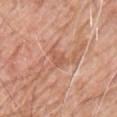Assessment: No biopsy was performed on this lesion — it was imaged during a full skin examination and was not determined to be concerning. Background: An algorithmic analysis of the crop reported an area of roughly 4.5 mm², an eccentricity of roughly 0.75, and a symmetry-axis asymmetry near 0.45. The software also gave border irregularity of about 4.5 on a 0–10 scale, internal color variation of about 2 on a 0–10 scale, and peripheral color asymmetry of about 0.5. The analysis additionally found a nevus-likeness score of about 0/100 and lesion-presence confidence of about 90/100. A 15 mm crop from a total-body photograph taken for skin-cancer surveillance. Longest diameter approximately 3 mm. The lesion is on the chest. This is a white-light tile. A male patient in their 60s.